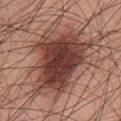This lesion was catalogued during total-body skin photography and was not selected for biopsy. The subject is a male aged around 60. Located on the chest. A 15 mm close-up tile from a total-body photography series done for melanoma screening.